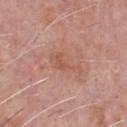<record>
<lesion_size>
  <long_diameter_mm_approx>4.0</long_diameter_mm_approx>
</lesion_size>
<site>front of the torso</site>
<lighting>white-light</lighting>
<patient>
  <sex>male</sex>
  <age_approx>60</age_approx>
</patient>
<automated_metrics>
  <cielab_L>55</cielab_L>
  <cielab_a>25</cielab_a>
  <cielab_b>29</cielab_b>
  <vs_skin_contrast_norm>5.5</vs_skin_contrast_norm>
  <border_irregularity_0_10>7.0</border_irregularity_0_10>
  <color_variation_0_10>1.0</color_variation_0_10>
  <peripheral_color_asymmetry>0.0</peripheral_color_asymmetry>
</automated_metrics>
<image>
  <source>total-body photography crop</source>
  <field_of_view_mm>15</field_of_view_mm>
</image>
</record>